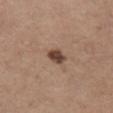The lesion was photographed on a routine skin check and not biopsied; there is no pathology result.
A close-up tile cropped from a whole-body skin photograph, about 15 mm across.
The lesion's longest dimension is about 2.5 mm.
Captured under white-light illumination.
Automated image analysis of the tile measured a border-irregularity rating of about 2/10, a color-variation rating of about 2.5/10, and peripheral color asymmetry of about 1. And it measured a nevus-likeness score of about 90/100 and lesion-presence confidence of about 100/100.
A female patient, in their mid-60s.
From the chest.